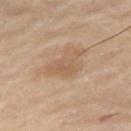Case summary:
- acquisition · total-body-photography crop, ~15 mm field of view
- anatomic site · the right thigh
- subject · male, roughly 65 years of age
- tile lighting · cross-polarized illumination
- automated lesion analysis · a mean CIELAB color near L≈57 a*≈18 b*≈31 and a lesion-to-skin contrast of about 5 (normalized; higher = more distinct); border irregularity of about 6.5 on a 0–10 scale, a color-variation rating of about 2/10, and peripheral color asymmetry of about 0.5